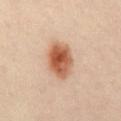Case summary:
– biopsy status: imaged on a skin check; not biopsied
– automated metrics: a normalized border contrast of about 10.5; peripheral color asymmetry of about 2
– patient: male, in their 50s
– tile lighting: cross-polarized
– anatomic site: the abdomen
– lesion size: ~4.5 mm (longest diameter)
– image: 15 mm crop, total-body photography